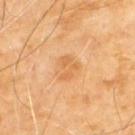| field | value |
|---|---|
| workup | imaged on a skin check; not biopsied |
| body site | the back |
| tile lighting | cross-polarized illumination |
| subject | male, roughly 70 years of age |
| image | 15 mm crop, total-body photography |